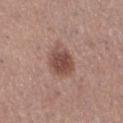This lesion was catalogued during total-body skin photography and was not selected for biopsy. The tile uses white-light illumination. The lesion is located on the left lower leg. The patient is a female roughly 30 years of age. A 15 mm close-up tile from a total-body photography series done for melanoma screening. Approximately 4.5 mm at its widest.